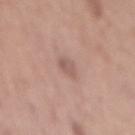Recorded during total-body skin imaging; not selected for excision or biopsy.
A 15 mm close-up tile from a total-body photography series done for melanoma screening.
A male patient aged around 60.
The lesion's longest dimension is about 2.5 mm.
From the back.